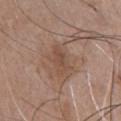| key | value |
|---|---|
| biopsy status | imaged on a skin check; not biopsied |
| image source | ~15 mm crop, total-body skin-cancer survey |
| subject | male, approximately 50 years of age |
| illumination | white-light illumination |
| diameter | about 3.5 mm |
| site | the chest |